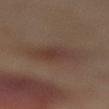The lesion was photographed on a routine skin check and not biopsied; there is no pathology result. A female patient, roughly 55 years of age. The lesion is located on the left lower leg. Cropped from a whole-body photographic skin survey; the tile spans about 15 mm.